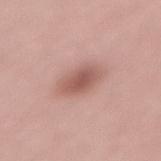Part of a total-body skin-imaging series; this lesion was reviewed on a skin check and was not flagged for biopsy. The lesion is located on the back. The lesion-visualizer software estimated a lesion color around L≈57 a*≈22 b*≈25 in CIELAB, about 11 CIELAB-L* units darker than the surrounding skin, and a lesion-to-skin contrast of about 7 (normalized; higher = more distinct). It also reported a border-irregularity index near 1.5/10 and a color-variation rating of about 3.5/10. A male subject in their mid-50s. About 4 mm across. The tile uses white-light illumination. Cropped from a whole-body photographic skin survey; the tile spans about 15 mm.